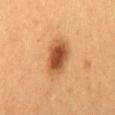No biopsy was performed on this lesion — it was imaged during a full skin examination and was not determined to be concerning. From the back. This image is a 15 mm lesion crop taken from a total-body photograph. About 4.5 mm across. Imaged with cross-polarized lighting. A female patient, aged 38 to 42.